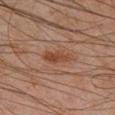The lesion was tiled from a total-body skin photograph and was not biopsied.
A 15 mm crop from a total-body photograph taken for skin-cancer surveillance.
The subject is a male aged approximately 45.
The lesion is on the leg.
Measured at roughly 3.5 mm in maximum diameter.